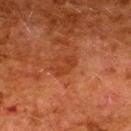follow-up: total-body-photography surveillance lesion; no biopsy
tile lighting: cross-polarized
size: about 3 mm
image-analysis metrics: an outline eccentricity of about 0.85 (0 = round, 1 = elongated) and a symmetry-axis asymmetry near 0.35; a mean CIELAB color near L≈34 a*≈27 b*≈33 and a normalized border contrast of about 5.5; internal color variation of about 1 on a 0–10 scale and a peripheral color-asymmetry measure near 0
patient: female, in their 50s
location: the upper back
image source: total-body-photography crop, ~15 mm field of view